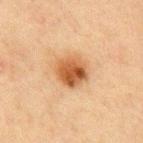Notes:
* workup: catalogued during a skin exam; not biopsied
* body site: the abdomen
* image: 15 mm crop, total-body photography
* patient: male, in their mid-70s
* lesion size: ~3.5 mm (longest diameter)